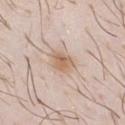Q: Was this lesion biopsied?
A: no biopsy performed (imaged during a skin exam)
Q: What is the anatomic site?
A: the chest
Q: What are the patient's age and sex?
A: male, aged 28–32
Q: How was this image acquired?
A: total-body-photography crop, ~15 mm field of view
Q: How large is the lesion?
A: ≈3 mm